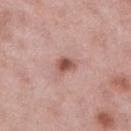This lesion was catalogued during total-body skin photography and was not selected for biopsy.
The recorded lesion diameter is about 3 mm.
Cropped from a whole-body photographic skin survey; the tile spans about 15 mm.
A female subject, roughly 50 years of age.
The lesion is on the right lower leg.
The tile uses white-light illumination.